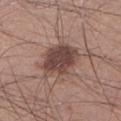Assessment: No biopsy was performed on this lesion — it was imaged during a full skin examination and was not determined to be concerning. Background: Imaged with white-light lighting. A 15 mm crop from a total-body photograph taken for skin-cancer surveillance. A male patient aged approximately 30. Longest diameter approximately 5 mm. From the leg.